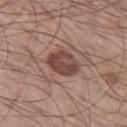workup: catalogued during a skin exam; not biopsied | TBP lesion metrics: about 12 CIELAB-L* units darker than the surrounding skin and a lesion-to-skin contrast of about 9.5 (normalized; higher = more distinct); a border-irregularity index near 1.5/10 and internal color variation of about 4 on a 0–10 scale | subject: male, aged 58 to 62 | image source: ~15 mm crop, total-body skin-cancer survey | location: the left thigh.